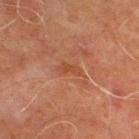Q: What kind of image is this?
A: ~15 mm tile from a whole-body skin photo
Q: Lesion location?
A: the right lower leg
Q: Automated lesion metrics?
A: a lesion color around L≈39 a*≈23 b*≈30 in CIELAB and roughly 5 lightness units darker than nearby skin
Q: Patient demographics?
A: male, roughly 70 years of age
Q: How large is the lesion?
A: ≈3 mm